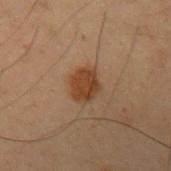Clinical impression: No biopsy was performed on this lesion — it was imaged during a full skin examination and was not determined to be concerning. Background: A 15 mm close-up tile from a total-body photography series done for melanoma screening. A male patient, aged approximately 55. Approximately 3.5 mm at its widest. The lesion-visualizer software estimated a lesion color around L≈33 a*≈17 b*≈28 in CIELAB, a lesion–skin lightness drop of about 8, and a normalized lesion–skin contrast near 9. The analysis additionally found a border-irregularity index near 1.5/10 and radial color variation of about 0.5. It also reported a lesion-detection confidence of about 100/100. On the left upper arm.